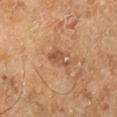{
  "biopsy_status": "not biopsied; imaged during a skin examination",
  "lesion_size": {
    "long_diameter_mm_approx": 3.0
  },
  "automated_metrics": {
    "cielab_L": 52,
    "cielab_a": 22,
    "cielab_b": 35,
    "vs_skin_darker_L": 9.0,
    "border_irregularity_0_10": 4.5,
    "color_variation_0_10": 1.5,
    "peripheral_color_asymmetry": 0.5,
    "nevus_likeness_0_100": 0,
    "lesion_detection_confidence_0_100": 100
  },
  "patient": {
    "sex": "male",
    "age_approx": 65
  },
  "image": {
    "source": "total-body photography crop",
    "field_of_view_mm": 15
  },
  "site": "right lower leg",
  "lighting": "cross-polarized"
}